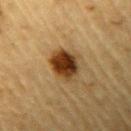Recorded during total-body skin imaging; not selected for excision or biopsy. Captured under cross-polarized illumination. The lesion is located on the left upper arm. A 15 mm close-up tile from a total-body photography series done for melanoma screening. The patient is a male in their mid- to late 80s or older. Approximately 4 mm at its widest. The lesion-visualizer software estimated an outline eccentricity of about 0.65 (0 = round, 1 = elongated) and two-axis asymmetry of about 0.2. The software also gave border irregularity of about 2 on a 0–10 scale, a color-variation rating of about 6.5/10, and radial color variation of about 2.